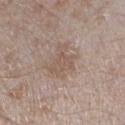image=~15 mm crop, total-body skin-cancer survey
anatomic site=the left lower leg
size=~4 mm (longest diameter)
TBP lesion metrics=a lesion area of about 8.5 mm² and a shape eccentricity near 0.65; a lesion–skin lightness drop of about 7 and a normalized border contrast of about 5.5; an automated nevus-likeness rating near 0 out of 100 and a detector confidence of about 100 out of 100 that the crop contains a lesion
subject=male, in their mid-40s
tile lighting=white-light illumination